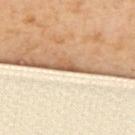Findings:
- notes — imaged on a skin check; not biopsied
- image — ~15 mm tile from a whole-body skin photo
- body site — the upper back
- lesion diameter — ≈2.5 mm
- automated lesion analysis — an outline eccentricity of about 0.95 (0 = round, 1 = elongated) and two-axis asymmetry of about 0.45
- patient — female, aged 53–57
- tile lighting — cross-polarized illumination